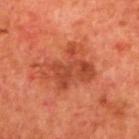Q: Was a biopsy performed?
A: total-body-photography surveillance lesion; no biopsy
Q: Patient demographics?
A: male, aged around 70
Q: What is the anatomic site?
A: the mid back
Q: What lighting was used for the tile?
A: cross-polarized
Q: How was this image acquired?
A: 15 mm crop, total-body photography